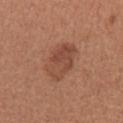notes = total-body-photography surveillance lesion; no biopsy | patient = female, aged approximately 40 | TBP lesion metrics = an area of roughly 13 mm²; an automated nevus-likeness rating near 25 out of 100 and a lesion-detection confidence of about 100/100 | anatomic site = the right upper arm | tile lighting = white-light | lesion diameter = ~5 mm (longest diameter) | imaging modality = 15 mm crop, total-body photography.